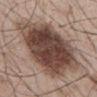No biopsy was performed on this lesion — it was imaged during a full skin examination and was not determined to be concerning. A region of skin cropped from a whole-body photographic capture, roughly 15 mm wide. The lesion is on the chest. A male subject, approximately 55 years of age.